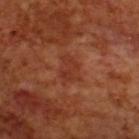Assessment: No biopsy was performed on this lesion — it was imaged during a full skin examination and was not determined to be concerning. Background: A male patient about 70 years old. A roughly 15 mm field-of-view crop from a total-body skin photograph. From the upper back.